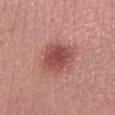– subject · male, approximately 20 years of age
– lighting · white-light
– lesion size · about 4 mm
– anatomic site · the leg
– imaging modality · total-body-photography crop, ~15 mm field of view
– image-analysis metrics · lesion-presence confidence of about 100/100